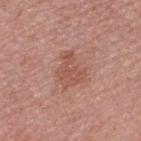Imaged during a routine full-body skin examination; the lesion was not biopsied and no histopathology is available.